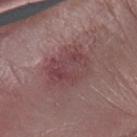Notes:
- biopsy status · no biopsy performed (imaged during a skin exam)
- image source · total-body-photography crop, ~15 mm field of view
- size · ≈6 mm
- automated metrics · a footprint of about 19 mm², an outline eccentricity of about 0.7 (0 = round, 1 = elongated), and a shape-asymmetry score of about 0.2 (0 = symmetric); a lesion color around L≈43 a*≈22 b*≈17 in CIELAB and a lesion–skin lightness drop of about 8; a border-irregularity rating of about 3/10, internal color variation of about 4.5 on a 0–10 scale, and a peripheral color-asymmetry measure near 1.5
- site · the left forearm
- subject · female, roughly 85 years of age or older